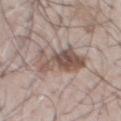Assessment: Captured during whole-body skin photography for melanoma surveillance; the lesion was not biopsied. Clinical summary: A lesion tile, about 15 mm wide, cut from a 3D total-body photograph. On the chest. A male patient, aged 68 to 72. Measured at roughly 5.5 mm in maximum diameter. An algorithmic analysis of the crop reported an outline eccentricity of about 0.8 (0 = round, 1 = elongated) and two-axis asymmetry of about 0.35.